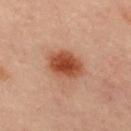<record>
  <site>chest</site>
  <patient>
    <sex>female</sex>
    <age_approx>50</age_approx>
  </patient>
  <image>
    <source>total-body photography crop</source>
    <field_of_view_mm>15</field_of_view_mm>
  </image>
</record>